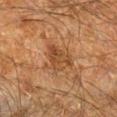Findings:
* biopsy status · imaged on a skin check; not biopsied
* illumination · cross-polarized
* imaging modality · 15 mm crop, total-body photography
* subject · male, aged approximately 60
* lesion size · ~4 mm (longest diameter)
* body site · the right lower leg